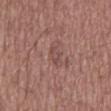notes=imaged on a skin check; not biopsied | automated metrics=a lesion area of about 2.5 mm², a shape eccentricity near 0.9, and two-axis asymmetry of about 0.45; a mean CIELAB color near L≈47 a*≈21 b*≈21 and a normalized lesion–skin contrast near 5; a border-irregularity index near 5.5/10, a color-variation rating of about 0/10, and radial color variation of about 0; an automated nevus-likeness rating near 0 out of 100 and lesion-presence confidence of about 80/100 | anatomic site=the mid back | patient=male, roughly 60 years of age | acquisition=~15 mm crop, total-body skin-cancer survey | diameter=about 2.5 mm | tile lighting=white-light illumination.